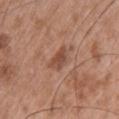<record>
  <biopsy_status>not biopsied; imaged during a skin examination</biopsy_status>
  <site>upper back</site>
  <patient>
    <sex>male</sex>
    <age_approx>50</age_approx>
  </patient>
  <image>
    <source>total-body photography crop</source>
    <field_of_view_mm>15</field_of_view_mm>
  </image>
</record>